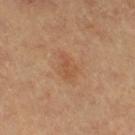Notes:
• patient — female, aged around 60
• imaging modality — total-body-photography crop, ~15 mm field of view
• lesion diameter — ≈3.5 mm
• location — the right thigh
• tile lighting — cross-polarized illumination